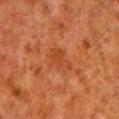Captured during whole-body skin photography for melanoma surveillance; the lesion was not biopsied.
The lesion-visualizer software estimated a mean CIELAB color near L≈36 a*≈25 b*≈32, roughly 5 lightness units darker than nearby skin, and a normalized border contrast of about 5. The analysis additionally found a nevus-likeness score of about 0/100.
The lesion is on the left lower leg.
A male subject aged around 80.
Imaged with cross-polarized lighting.
About 4 mm across.
A region of skin cropped from a whole-body photographic capture, roughly 15 mm wide.